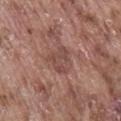body site = the back
subject = male, aged approximately 75
lesion size = ≈3.5 mm
image-analysis metrics = a footprint of about 6 mm², a shape eccentricity near 0.75, and two-axis asymmetry of about 0.35; a mean CIELAB color near L≈47 a*≈22 b*≈24, about 8 CIELAB-L* units darker than the surrounding skin, and a normalized lesion–skin contrast near 6; border irregularity of about 3.5 on a 0–10 scale, a color-variation rating of about 3/10, and radial color variation of about 1
imaging modality = ~15 mm tile from a whole-body skin photo
tile lighting = white-light illumination